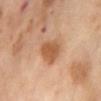{
  "biopsy_status": "not biopsied; imaged during a skin examination",
  "image": {
    "source": "total-body photography crop",
    "field_of_view_mm": 15
  },
  "site": "front of the torso",
  "lesion_size": {
    "long_diameter_mm_approx": 4.0
  },
  "patient": {
    "sex": "female",
    "age_approx": 55
  }
}